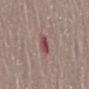<record>
<biopsy_status>not biopsied; imaged during a skin examination</biopsy_status>
<image>
  <source>total-body photography crop</source>
  <field_of_view_mm>15</field_of_view_mm>
</image>
<patient>
  <sex>female</sex>
  <age_approx>70</age_approx>
</patient>
<lesion_size>
  <long_diameter_mm_approx>2.5</long_diameter_mm_approx>
</lesion_size>
<site>mid back</site>
</record>